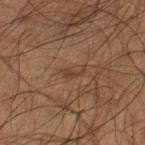The lesion was tiled from a total-body skin photograph and was not biopsied.
The lesion's longest dimension is about 2.5 mm.
This is a cross-polarized tile.
This image is a 15 mm lesion crop taken from a total-body photograph.
On the right thigh.
The subject is a male about 50 years old.